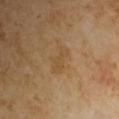The lesion was tiled from a total-body skin photograph and was not biopsied. Imaged with cross-polarized lighting. A region of skin cropped from a whole-body photographic capture, roughly 15 mm wide. A male patient approximately 65 years of age. About 3.5 mm across.